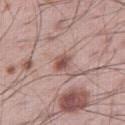Q: Was a biopsy performed?
A: imaged on a skin check; not biopsied
Q: What kind of image is this?
A: ~15 mm tile from a whole-body skin photo
Q: Where on the body is the lesion?
A: the right thigh
Q: Patient demographics?
A: male, aged around 60
Q: Lesion size?
A: ≈2.5 mm
Q: Automated lesion metrics?
A: a footprint of about 4 mm², an outline eccentricity of about 0.65 (0 = round, 1 = elongated), and two-axis asymmetry of about 0.25; a normalized border contrast of about 8.5